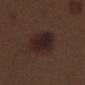Q: Lesion location?
A: the left thigh
Q: What is the imaging modality?
A: ~15 mm crop, total-body skin-cancer survey
Q: What are the patient's age and sex?
A: male, aged approximately 70